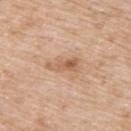Recorded during total-body skin imaging; not selected for excision or biopsy.
Approximately 3.5 mm at its widest.
The patient is a male aged 63–67.
This image is a 15 mm lesion crop taken from a total-body photograph.
Captured under white-light illumination.
On the back.
The lesion-visualizer software estimated a lesion area of about 5 mm², an eccentricity of roughly 0.9, and a shape-asymmetry score of about 0.4 (0 = symmetric). The software also gave a lesion–skin lightness drop of about 10 and a normalized lesion–skin contrast near 6.5.